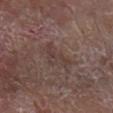  biopsy_status: not biopsied; imaged during a skin examination
  lighting: white-light
  automated_metrics:
    color_variation_0_10: 1.5
    peripheral_color_asymmetry: 0.5
    lesion_detection_confidence_0_100: 95
  lesion_size:
    long_diameter_mm_approx: 3.5
  image:
    source: total-body photography crop
    field_of_view_mm: 15
  patient:
    sex: male
    age_approx: 80
  site: right arm